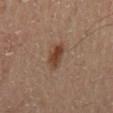follow-up: catalogued during a skin exam; not biopsied | TBP lesion metrics: a footprint of about 5.5 mm², an eccentricity of roughly 0.85, and two-axis asymmetry of about 0.25; an average lesion color of about L≈40 a*≈19 b*≈27 (CIELAB), roughly 10 lightness units darker than nearby skin, and a lesion-to-skin contrast of about 9 (normalized; higher = more distinct); border irregularity of about 3 on a 0–10 scale, a color-variation rating of about 3.5/10, and a peripheral color-asymmetry measure near 1.5; an automated nevus-likeness rating near 95 out of 100 and lesion-presence confidence of about 100/100 | subject: male, roughly 55 years of age | site: the mid back | tile lighting: cross-polarized illumination | image source: ~15 mm tile from a whole-body skin photo.